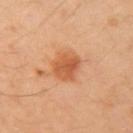This lesion was catalogued during total-body skin photography and was not selected for biopsy.
A male patient in their mid-50s.
The lesion's longest dimension is about 4 mm.
A region of skin cropped from a whole-body photographic capture, roughly 15 mm wide.
This is a cross-polarized tile.
On the arm.